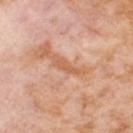Clinical impression:
Captured during whole-body skin photography for melanoma surveillance; the lesion was not biopsied.
Clinical summary:
The lesion is on the right thigh. A female patient approximately 55 years of age. A region of skin cropped from a whole-body photographic capture, roughly 15 mm wide.